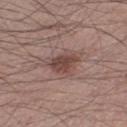Case summary:
* biopsy status: imaged on a skin check; not biopsied
* site: the right thigh
* acquisition: ~15 mm crop, total-body skin-cancer survey
* patient: male, aged around 55